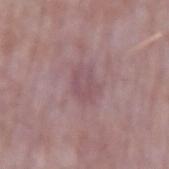{
  "biopsy_status": "not biopsied; imaged during a skin examination",
  "site": "right forearm",
  "patient": {
    "sex": "male",
    "age_approx": 65
  },
  "image": {
    "source": "total-body photography crop",
    "field_of_view_mm": 15
  }
}